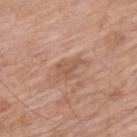Q: Is there a histopathology result?
A: no biopsy performed (imaged during a skin exam)
Q: What is the anatomic site?
A: the back
Q: What are the patient's age and sex?
A: male, about 60 years old
Q: What is the imaging modality?
A: 15 mm crop, total-body photography